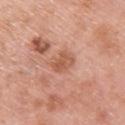{"biopsy_status": "not biopsied; imaged during a skin examination", "lesion_size": {"long_diameter_mm_approx": 3.0}, "automated_metrics": {"area_mm2_approx": 6.5, "shape_asymmetry": 0.2}, "site": "upper back", "image": {"source": "total-body photography crop", "field_of_view_mm": 15}, "lighting": "white-light", "patient": {"sex": "male", "age_approx": 60}}